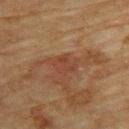  biopsy_status: not biopsied; imaged during a skin examination
  image:
    source: total-body photography crop
    field_of_view_mm: 15
  site: upper back
  lesion_size:
    long_diameter_mm_approx: 3.0
  lighting: cross-polarized
  patient:
    sex: female
    age_approx: 80